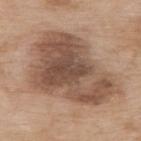Part of a total-body skin-imaging series; this lesion was reviewed on a skin check and was not flagged for biopsy. Captured under white-light illumination. A 15 mm close-up extracted from a 3D total-body photography capture. The lesion is on the upper back. The patient is a female aged 73–77.